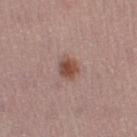| feature | finding |
|---|---|
| subject | female, aged approximately 25 |
| imaging modality | total-body-photography crop, ~15 mm field of view |
| illumination | white-light |
| lesion diameter | about 3 mm |
| location | the right thigh |
| image-analysis metrics | an eccentricity of roughly 0.6 and a symmetry-axis asymmetry near 0.15 |